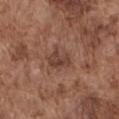<lesion>
<biopsy_status>not biopsied; imaged during a skin examination</biopsy_status>
<image>
  <source>total-body photography crop</source>
  <field_of_view_mm>15</field_of_view_mm>
</image>
<site>chest</site>
<patient>
  <sex>male</sex>
  <age_approx>75</age_approx>
</patient>
</lesion>